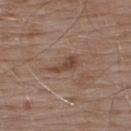workup: total-body-photography surveillance lesion; no biopsy
patient: male, approximately 55 years of age
image source: total-body-photography crop, ~15 mm field of view
site: the upper back
diameter: ≈4 mm
illumination: white-light illumination